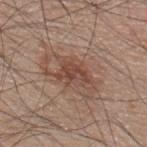patient:
  sex: male
  age_approx: 45
lesion_size:
  long_diameter_mm_approx: 5.0
automated_metrics:
  border_irregularity_0_10: 4.0
  peripheral_color_asymmetry: 1.0
lighting: white-light
site: upper back
image:
  source: total-body photography crop
  field_of_view_mm: 15